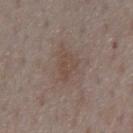Clinical impression: Recorded during total-body skin imaging; not selected for excision or biopsy. Image and clinical context: A lesion tile, about 15 mm wide, cut from a 3D total-body photograph. A male subject, aged approximately 75. The lesion is on the chest.